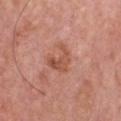Q: What lighting was used for the tile?
A: white-light illumination
Q: Who is the patient?
A: male, about 80 years old
Q: Lesion size?
A: ≈3.5 mm
Q: What is the anatomic site?
A: the chest
Q: What is the imaging modality?
A: 15 mm crop, total-body photography